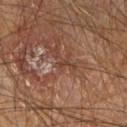{"lighting": "cross-polarized", "image": {"source": "total-body photography crop", "field_of_view_mm": 15}, "automated_metrics": {"color_variation_0_10": 0.0, "lesion_detection_confidence_0_100": 45}, "patient": {"sex": "male", "age_approx": 65}, "lesion_size": {"long_diameter_mm_approx": 1.0}, "site": "right upper arm"}